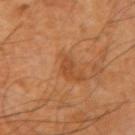Q: Is there a histopathology result?
A: catalogued during a skin exam; not biopsied
Q: Patient demographics?
A: male, aged 58 to 62
Q: Lesion size?
A: ≈3 mm
Q: What is the anatomic site?
A: the right upper arm
Q: What kind of image is this?
A: ~15 mm crop, total-body skin-cancer survey